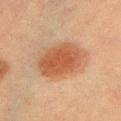The lesion was tiled from a total-body skin photograph and was not biopsied. The lesion is located on the chest. Longest diameter approximately 6.5 mm. The tile uses cross-polarized illumination. Cropped from a whole-body photographic skin survey; the tile spans about 15 mm. The subject is a female roughly 55 years of age.